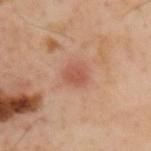Assessment: Recorded during total-body skin imaging; not selected for excision or biopsy. Clinical summary: The recorded lesion diameter is about 2.5 mm. A male subject, approximately 55 years of age. The total-body-photography lesion software estimated an area of roughly 5 mm² and an outline eccentricity of about 0.5 (0 = round, 1 = elongated). The analysis additionally found a mean CIELAB color near L≈54 a*≈26 b*≈31, a lesion–skin lightness drop of about 9, and a normalized border contrast of about 6. It also reported a color-variation rating of about 2/10 and peripheral color asymmetry of about 0.5. The analysis additionally found an automated nevus-likeness rating near 10 out of 100 and a lesion-detection confidence of about 100/100. From the upper back. Captured under cross-polarized illumination. A roughly 15 mm field-of-view crop from a total-body skin photograph.